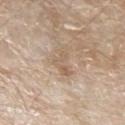anatomic site: the left forearm | tile lighting: white-light | lesion diameter: about 3.5 mm | subject: male, in their 80s | automated lesion analysis: a lesion area of about 4.5 mm² and an eccentricity of roughly 0.85; a color-variation rating of about 2/10 and a peripheral color-asymmetry measure near 0; a classifier nevus-likeness of about 0/100 and a detector confidence of about 90 out of 100 that the crop contains a lesion | imaging modality: 15 mm crop, total-body photography.